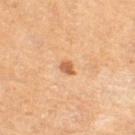Q: Is there a histopathology result?
A: catalogued during a skin exam; not biopsied